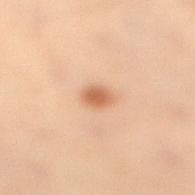workup: imaged on a skin check; not biopsied
anatomic site: the right lower leg
subject: female, aged approximately 50
lighting: cross-polarized illumination
image: 15 mm crop, total-body photography